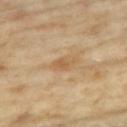notes — total-body-photography surveillance lesion; no biopsy | imaging modality — total-body-photography crop, ~15 mm field of view | lesion diameter — ≈2.5 mm | subject — female, roughly 75 years of age | automated metrics — a footprint of about 3.5 mm², an outline eccentricity of about 0.8 (0 = round, 1 = elongated), and two-axis asymmetry of about 0.3; a lesion color around L≈58 a*≈17 b*≈38 in CIELAB, a lesion–skin lightness drop of about 7, and a normalized border contrast of about 5.5; a detector confidence of about 100 out of 100 that the crop contains a lesion | anatomic site — the chest.